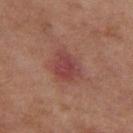Recorded during total-body skin imaging; not selected for excision or biopsy.
Captured under cross-polarized illumination.
The patient is a female aged around 60.
Measured at roughly 3.5 mm in maximum diameter.
Cropped from a whole-body photographic skin survey; the tile spans about 15 mm.
The lesion is on the left thigh.